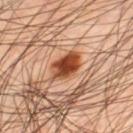The lesion was tiled from a total-body skin photograph and was not biopsied.
The patient is a male aged around 45.
Automated image analysis of the tile measured a lesion color around L≈44 a*≈26 b*≈35 in CIELAB, a lesion–skin lightness drop of about 17, and a normalized border contrast of about 12.5. And it measured border irregularity of about 2 on a 0–10 scale, a within-lesion color-variation index near 5.5/10, and radial color variation of about 1.5.
Measured at roughly 4 mm in maximum diameter.
A 15 mm close-up tile from a total-body photography series done for melanoma screening.
Located on the leg.
Imaged with cross-polarized lighting.